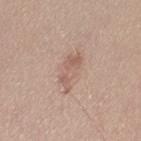Impression:
The lesion was tiled from a total-body skin photograph and was not biopsied.
Image and clinical context:
The lesion is located on the left thigh. Longest diameter approximately 4.5 mm. Cropped from a whole-body photographic skin survey; the tile spans about 15 mm. A female subject, aged approximately 45.